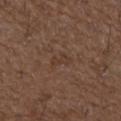Assessment: No biopsy was performed on this lesion — it was imaged during a full skin examination and was not determined to be concerning. Background: Measured at roughly 2.5 mm in maximum diameter. The patient is a male about 50 years old. Imaged with white-light lighting. Cropped from a whole-body photographic skin survey; the tile spans about 15 mm. On the left forearm.